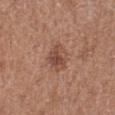| key | value |
|---|---|
| workup | total-body-photography surveillance lesion; no biopsy |
| TBP lesion metrics | a footprint of about 6.5 mm² and a shape eccentricity near 0.5; a lesion-to-skin contrast of about 7 (normalized; higher = more distinct); an automated nevus-likeness rating near 65 out of 100 and a detector confidence of about 100 out of 100 that the crop contains a lesion |
| tile lighting | white-light |
| location | the left lower leg |
| imaging modality | total-body-photography crop, ~15 mm field of view |
| patient | female, aged around 40 |